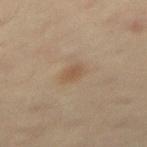Case summary:
- notes: imaged on a skin check; not biopsied
- subject: male, aged 58–62
- location: the right thigh
- tile lighting: cross-polarized
- size: ~3 mm (longest diameter)
- imaging modality: ~15 mm tile from a whole-body skin photo